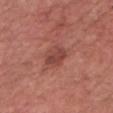Part of a total-body skin-imaging series; this lesion was reviewed on a skin check and was not flagged for biopsy.
Automated tile analysis of the lesion measured a footprint of about 5 mm², an outline eccentricity of about 0.8 (0 = round, 1 = elongated), and two-axis asymmetry of about 0.2. The software also gave a border-irregularity index near 2/10, a within-lesion color-variation index near 2.5/10, and radial color variation of about 1. It also reported a nevus-likeness score of about 50/100 and a detector confidence of about 100 out of 100 that the crop contains a lesion.
About 3 mm across.
Cropped from a whole-body photographic skin survey; the tile spans about 15 mm.
The subject is a male aged 78 to 82.
The lesion is located on the chest.